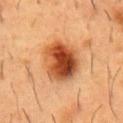Clinical impression:
Captured during whole-body skin photography for melanoma surveillance; the lesion was not biopsied.
Clinical summary:
From the chest. An algorithmic analysis of the crop reported an area of roughly 18 mm², an outline eccentricity of about 0.55 (0 = round, 1 = elongated), and two-axis asymmetry of about 0.15. It also reported border irregularity of about 1.5 on a 0–10 scale, a color-variation rating of about 10/10, and a peripheral color-asymmetry measure near 3. A male patient in their mid- to late 50s. Cropped from a whole-body photographic skin survey; the tile spans about 15 mm. The lesion's longest dimension is about 5.5 mm. The tile uses cross-polarized illumination.